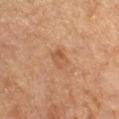This lesion was catalogued during total-body skin photography and was not selected for biopsy.
The tile uses cross-polarized illumination.
The lesion is on the leg.
The subject is a female in their mid-50s.
A 15 mm close-up extracted from a 3D total-body photography capture.
Approximately 2.5 mm at its widest.